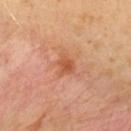This lesion was catalogued during total-body skin photography and was not selected for biopsy.
A 15 mm close-up extracted from a 3D total-body photography capture.
Captured under cross-polarized illumination.
Automated image analysis of the tile measured a lesion area of about 4.5 mm², an eccentricity of roughly 0.5, and two-axis asymmetry of about 0.3. It also reported an average lesion color of about L≈55 a*≈28 b*≈37 (CIELAB).
Located on the upper back.
The subject is a male aged 38 to 42.